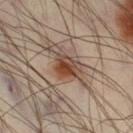notes — imaged on a skin check; not biopsied | subject — male, in their 40s | acquisition — ~15 mm crop, total-body skin-cancer survey | site — the leg.